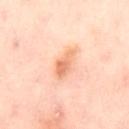Case summary:
– follow-up — imaged on a skin check; not biopsied
– lighting — cross-polarized
– diameter — ~3.5 mm (longest diameter)
– site — the leg
– automated lesion analysis — a footprint of about 6 mm², a shape eccentricity near 0.85, and a shape-asymmetry score of about 0.25 (0 = symmetric); an average lesion color of about L≈75 a*≈26 b*≈39 (CIELAB); border irregularity of about 2.5 on a 0–10 scale and internal color variation of about 4.5 on a 0–10 scale; a nevus-likeness score of about 60/100 and a lesion-detection confidence of about 100/100
– imaging modality — ~15 mm tile from a whole-body skin photo
– patient — female, aged 53 to 57